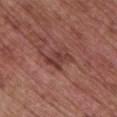Notes:
* notes: imaged on a skin check; not biopsied
* imaging modality: ~15 mm tile from a whole-body skin photo
* size: ≈3.5 mm
* body site: the chest
* subject: female, roughly 65 years of age